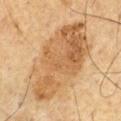Context:
The subject is a male in their mid- to late 60s. The lesion is on the chest. Captured under cross-polarized illumination. A 15 mm close-up extracted from a 3D total-body photography capture. Automated image analysis of the tile measured an area of roughly 48 mm² and an outline eccentricity of about 0.85 (0 = round, 1 = elongated). The software also gave a color-variation rating of about 6/10 and peripheral color asymmetry of about 2.5.